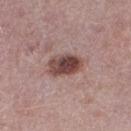  biopsy_status: not biopsied; imaged during a skin examination
  lesion_size:
    long_diameter_mm_approx: 4.0
  lighting: white-light
  site: right thigh
  patient:
    sex: male
    age_approx: 40
  image:
    source: total-body photography crop
    field_of_view_mm: 15
  automated_metrics:
    shape_asymmetry: 0.15
    cielab_L: 45
    cielab_a: 20
    cielab_b: 21
    vs_skin_darker_L: 15.0